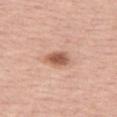Assessment:
This lesion was catalogued during total-body skin photography and was not selected for biopsy.
Image and clinical context:
From the right thigh. Cropped from a whole-body photographic skin survey; the tile spans about 15 mm. A female patient, aged 53 to 57. The tile uses white-light illumination.